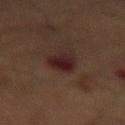workup: no biopsy performed (imaged during a skin exam)
TBP lesion metrics: border irregularity of about 2.5 on a 0–10 scale and a within-lesion color-variation index near 4.5/10
imaging modality: ~15 mm crop, total-body skin-cancer survey
location: the mid back
tile lighting: cross-polarized
lesion size: ≈3.5 mm
patient: male, aged 58 to 62